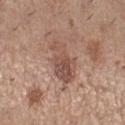notes = catalogued during a skin exam; not biopsied
size = ~5.5 mm (longest diameter)
location = the right lower leg
illumination = white-light
automated lesion analysis = border irregularity of about 4.5 on a 0–10 scale and a color-variation rating of about 3.5/10
patient = female, in their 30s
acquisition = 15 mm crop, total-body photography